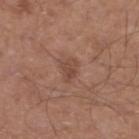| field | value |
|---|---|
| workup | imaged on a skin check; not biopsied |
| body site | the left lower leg |
| acquisition | total-body-photography crop, ~15 mm field of view |
| lesion diameter | ≈2.5 mm |
| illumination | white-light illumination |
| subject | male, aged 48–52 |